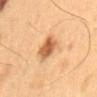<lesion>
<lighting>cross-polarized</lighting>
<patient>
  <sex>male</sex>
  <age_approx>60</age_approx>
</patient>
<lesion_size>
  <long_diameter_mm_approx>3.5</long_diameter_mm_approx>
</lesion_size>
<automated_metrics>
  <eccentricity>0.65</eccentricity>
  <shape_asymmetry>0.25</shape_asymmetry>
  <cielab_L>60</cielab_L>
  <cielab_a>24</cielab_a>
  <cielab_b>40</cielab_b>
  <vs_skin_darker_L>15.0</vs_skin_darker_L>
  <vs_skin_contrast_norm>9.0</vs_skin_contrast_norm>
</automated_metrics>
<image>
  <source>total-body photography crop</source>
  <field_of_view_mm>15</field_of_view_mm>
</image>
<site>lower back</site>
</lesion>